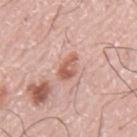workup: no biopsy performed (imaged during a skin exam) | diameter: ~3.5 mm (longest diameter) | patient: male, aged 73–77 | imaging modality: ~15 mm tile from a whole-body skin photo | anatomic site: the mid back | illumination: white-light illumination | automated metrics: an area of roughly 5 mm² and a shape-asymmetry score of about 0.25 (0 = symmetric); a mean CIELAB color near L≈60 a*≈24 b*≈28 and a lesion–skin lightness drop of about 11; a classifier nevus-likeness of about 0/100 and a lesion-detection confidence of about 100/100.